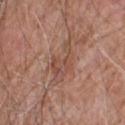Captured during whole-body skin photography for melanoma surveillance; the lesion was not biopsied.
On the upper back.
A 15 mm close-up extracted from a 3D total-body photography capture.
A male subject, aged around 80.